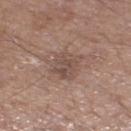| field | value |
|---|---|
| follow-up | catalogued during a skin exam; not biopsied |
| imaging modality | total-body-photography crop, ~15 mm field of view |
| diameter | about 3.5 mm |
| site | the left lower leg |
| automated lesion analysis | an average lesion color of about L≈49 a*≈17 b*≈23 (CIELAB), about 8 CIELAB-L* units darker than the surrounding skin, and a lesion-to-skin contrast of about 6 (normalized; higher = more distinct); a border-irregularity index near 2/10, a color-variation rating of about 3/10, and a peripheral color-asymmetry measure near 1 |
| tile lighting | white-light illumination |
| subject | male, approximately 65 years of age |